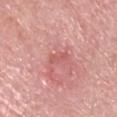Clinical impression: Recorded during total-body skin imaging; not selected for excision or biopsy. Image and clinical context: A close-up tile cropped from a whole-body skin photograph, about 15 mm across. On the head or neck. A male patient approximately 85 years of age or older.